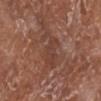biopsy status: imaged on a skin check; not biopsied
subject: female, aged 73–77
automated metrics: a lesion–skin lightness drop of about 6 and a normalized border contrast of about 5; border irregularity of about 6 on a 0–10 scale and a peripheral color-asymmetry measure near 0.5; lesion-presence confidence of about 70/100
lesion diameter: about 5 mm
site: the leg
image: 15 mm crop, total-body photography
tile lighting: white-light illumination